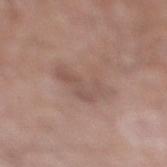Notes:
• patient · male, aged around 75
• acquisition · ~15 mm tile from a whole-body skin photo
• body site · the right lower leg
• automated metrics · a lesion color around L≈53 a*≈17 b*≈24 in CIELAB, roughly 7 lightness units darker than nearby skin, and a normalized border contrast of about 5